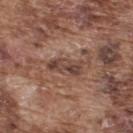Imaged during a routine full-body skin examination; the lesion was not biopsied and no histopathology is available. Cropped from a total-body skin-imaging series; the visible field is about 15 mm. The lesion is located on the upper back. The subject is a male in their mid-70s.